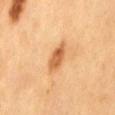<case>
<biopsy_status>not biopsied; imaged during a skin examination</biopsy_status>
<patient>
  <sex>male</sex>
  <age_approx>60</age_approx>
</patient>
<lighting>cross-polarized</lighting>
<site>back</site>
<image>
  <source>total-body photography crop</source>
  <field_of_view_mm>15</field_of_view_mm>
</image>
</case>